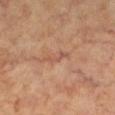Impression:
Part of a total-body skin-imaging series; this lesion was reviewed on a skin check and was not flagged for biopsy.
Background:
The lesion is located on the left lower leg. A female subject, aged 58 to 62. A close-up tile cropped from a whole-body skin photograph, about 15 mm across. The total-body-photography lesion software estimated a lesion area of about 3.5 mm², a shape eccentricity near 0.95, and a shape-asymmetry score of about 0.45 (0 = symmetric). The software also gave a mean CIELAB color near L≈50 a*≈21 b*≈28 and about 6 CIELAB-L* units darker than the surrounding skin. And it measured a border-irregularity rating of about 5.5/10, a color-variation rating of about 0/10, and a peripheral color-asymmetry measure near 0. The software also gave an automated nevus-likeness rating near 0 out of 100 and lesion-presence confidence of about 70/100. Captured under cross-polarized illumination.